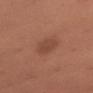biopsy_status: not biopsied; imaged during a skin examination
lesion_size:
  long_diameter_mm_approx: 3.0
image:
  source: total-body photography crop
  field_of_view_mm: 15
site: left upper arm
patient:
  sex: female
  age_approx: 50